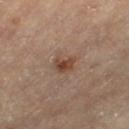{"biopsy_status": "not biopsied; imaged during a skin examination", "image": {"source": "total-body photography crop", "field_of_view_mm": 15}, "patient": {"sex": "male", "age_approx": "85+"}, "site": "left thigh", "lesion_size": {"long_diameter_mm_approx": 2.5}, "automated_metrics": {"area_mm2_approx": 4.5, "eccentricity": 0.65, "cielab_L": 42, "cielab_a": 18, "cielab_b": 27, "vs_skin_darker_L": 9.0, "vs_skin_contrast_norm": 8.0, "border_irregularity_0_10": 3.5, "nevus_likeness_0_100": 85, "lesion_detection_confidence_0_100": 100}, "lighting": "cross-polarized"}